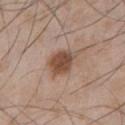Captured during whole-body skin photography for melanoma surveillance; the lesion was not biopsied.
The lesion is on the chest.
Cropped from a total-body skin-imaging series; the visible field is about 15 mm.
A male subject, approximately 50 years of age.
The tile uses white-light illumination.